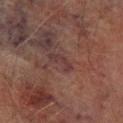A male subject, in their mid- to late 70s.
From the right lower leg.
About 3.5 mm across.
A close-up tile cropped from a whole-body skin photograph, about 15 mm across.
Automated image analysis of the tile measured a lesion area of about 3 mm² and two-axis asymmetry of about 0.5. The analysis additionally found a lesion color around L≈28 a*≈17 b*≈17 in CIELAB and a normalized lesion–skin contrast near 6.5. And it measured internal color variation of about 0 on a 0–10 scale. The analysis additionally found lesion-presence confidence of about 55/100.